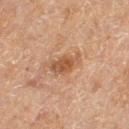Clinical impression:
Recorded during total-body skin imaging; not selected for excision or biopsy.
Image and clinical context:
The lesion is on the left thigh. A female subject in their mid- to late 50s. The recorded lesion diameter is about 4 mm. A roughly 15 mm field-of-view crop from a total-body skin photograph. The tile uses cross-polarized illumination. An algorithmic analysis of the crop reported an average lesion color of about L≈47 a*≈19 b*≈31 (CIELAB), about 9 CIELAB-L* units darker than the surrounding skin, and a normalized lesion–skin contrast near 7. The software also gave a border-irregularity index near 3.5/10, internal color variation of about 4 on a 0–10 scale, and peripheral color asymmetry of about 1.5. And it measured a nevus-likeness score of about 25/100 and a lesion-detection confidence of about 100/100.